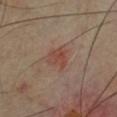image source = 15 mm crop, total-body photography
lesion size = about 2.5 mm
site = the chest
image-analysis metrics = a mean CIELAB color near L≈43 a*≈22 b*≈26, a lesion–skin lightness drop of about 7, and a normalized lesion–skin contrast near 6; an automated nevus-likeness rating near 5 out of 100 and lesion-presence confidence of about 100/100
patient = male, approximately 45 years of age
illumination = cross-polarized illumination